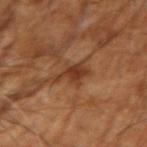Q: Was a biopsy performed?
A: imaged on a skin check; not biopsied
Q: What is the imaging modality?
A: 15 mm crop, total-body photography
Q: Who is the patient?
A: male, aged 53–57
Q: What is the anatomic site?
A: the right upper arm